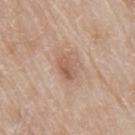imaging modality: ~15 mm crop, total-body skin-cancer survey
lesion size: ≈3 mm
location: the front of the torso
patient: male, aged 78–82
automated metrics: a lesion area of about 5.5 mm² and a shape eccentricity near 0.75; internal color variation of about 5 on a 0–10 scale and radial color variation of about 2; a lesion-detection confidence of about 100/100